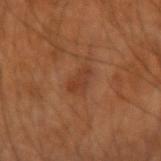* follow-up — total-body-photography surveillance lesion; no biopsy
* tile lighting — cross-polarized
* TBP lesion metrics — an eccentricity of roughly 0.85 and a shape-asymmetry score of about 0.35 (0 = symmetric); a lesion color around L≈39 a*≈23 b*≈32 in CIELAB, roughly 7 lightness units darker than nearby skin, and a normalized lesion–skin contrast near 6
* body site — the arm
* image source — ~15 mm tile from a whole-body skin photo
* patient — male, aged 48 to 52